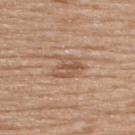Q: Lesion location?
A: the upper back
Q: Who is the patient?
A: female, aged 48–52
Q: What is the lesion's diameter?
A: ≈3.5 mm
Q: What kind of image is this?
A: ~15 mm tile from a whole-body skin photo
Q: What lighting was used for the tile?
A: white-light illumination